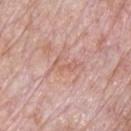<record>
<biopsy_status>not biopsied; imaged during a skin examination</biopsy_status>
<image>
  <source>total-body photography crop</source>
  <field_of_view_mm>15</field_of_view_mm>
</image>
<site>chest</site>
<lighting>white-light</lighting>
<patient>
  <sex>male</sex>
  <age_approx>70</age_approx>
</patient>
</record>